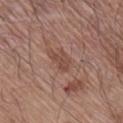follow-up = no biopsy performed (imaged during a skin exam); anatomic site = the right lower leg; patient = male, in their mid-60s; image = 15 mm crop, total-body photography.